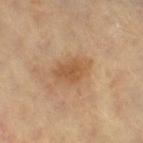Part of a total-body skin-imaging series; this lesion was reviewed on a skin check and was not flagged for biopsy. The lesion is on the right thigh. The patient is a female about 70 years old. A close-up tile cropped from a whole-body skin photograph, about 15 mm across. This is a cross-polarized tile.